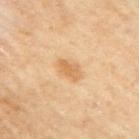Impression: Imaged during a routine full-body skin examination; the lesion was not biopsied and no histopathology is available. Image and clinical context: A female subject, approximately 60 years of age. Imaged with cross-polarized lighting. The total-body-photography lesion software estimated an area of roughly 4 mm², a shape eccentricity near 0.85, and a shape-asymmetry score of about 0.3 (0 = symmetric). The analysis additionally found border irregularity of about 3 on a 0–10 scale, a within-lesion color-variation index near 2/10, and radial color variation of about 1. Measured at roughly 3 mm in maximum diameter. The lesion is located on the right upper arm. Cropped from a whole-body photographic skin survey; the tile spans about 15 mm.